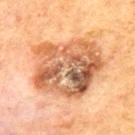| feature | finding |
|---|---|
| workup | total-body-photography surveillance lesion; no biopsy |
| illumination | cross-polarized illumination |
| body site | the upper back |
| patient | male, aged 68 to 72 |
| lesion size | ≈8.5 mm |
| automated lesion analysis | a lesion color around L≈50 a*≈20 b*≈32 in CIELAB and roughly 13 lightness units darker than nearby skin; a classifier nevus-likeness of about 20/100 and a lesion-detection confidence of about 100/100 |
| image source | ~15 mm crop, total-body skin-cancer survey |